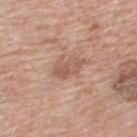The lesion was tiled from a total-body skin photograph and was not biopsied.
Automated tile analysis of the lesion measured an area of roughly 5 mm² and two-axis asymmetry of about 0.25.
The recorded lesion diameter is about 3.5 mm.
Imaged with white-light lighting.
From the upper back.
A region of skin cropped from a whole-body photographic capture, roughly 15 mm wide.
The patient is a male in their mid- to late 60s.